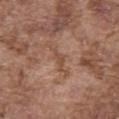* follow-up · catalogued during a skin exam; not biopsied
* patient · male, aged approximately 75
* lighting · white-light illumination
* diameter · ~3 mm (longest diameter)
* anatomic site · the abdomen
* image · ~15 mm crop, total-body skin-cancer survey